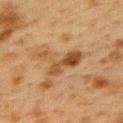Clinical impression: Imaged during a routine full-body skin examination; the lesion was not biopsied and no histopathology is available. Image and clinical context: Approximately 5.5 mm at its widest. This is a cross-polarized tile. The lesion is located on the upper back. A female patient, approximately 40 years of age. A region of skin cropped from a whole-body photographic capture, roughly 15 mm wide.